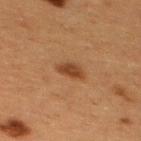Q: Was this lesion biopsied?
A: no biopsy performed (imaged during a skin exam)
Q: Lesion size?
A: ≈3 mm
Q: Where on the body is the lesion?
A: the upper back
Q: Automated lesion metrics?
A: a lesion color around L≈35 a*≈20 b*≈31 in CIELAB and a lesion–skin lightness drop of about 10; a classifier nevus-likeness of about 95/100 and lesion-presence confidence of about 100/100
Q: How was this image acquired?
A: 15 mm crop, total-body photography
Q: Illumination type?
A: cross-polarized
Q: Patient demographics?
A: female, aged 38–42